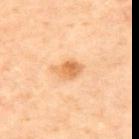Part of a total-body skin-imaging series; this lesion was reviewed on a skin check and was not flagged for biopsy. From the back. A female subject aged 58–62. Captured under cross-polarized illumination. About 3.5 mm across. A 15 mm crop from a total-body photograph taken for skin-cancer surveillance.